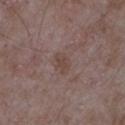Assessment: Imaged during a routine full-body skin examination; the lesion was not biopsied and no histopathology is available. Clinical summary: On the arm. The lesion-visualizer software estimated a footprint of about 3.5 mm² and two-axis asymmetry of about 0.35. The software also gave a border-irregularity rating of about 3/10 and a peripheral color-asymmetry measure near 0.5. The analysis additionally found a classifier nevus-likeness of about 0/100. This is a white-light tile. The recorded lesion diameter is about 2.5 mm. A male subject in their mid- to late 60s. A region of skin cropped from a whole-body photographic capture, roughly 15 mm wide.